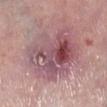<lesion>
<biopsy_status>not biopsied; imaged during a skin examination</biopsy_status>
<lighting>white-light</lighting>
<patient>
  <sex>male</sex>
  <age_approx>75</age_approx>
</patient>
<site>right lower leg</site>
<image>
  <source>total-body photography crop</source>
  <field_of_view_mm>15</field_of_view_mm>
</image>
</lesion>